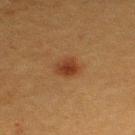Clinical impression: Recorded during total-body skin imaging; not selected for excision or biopsy. Acquisition and patient details: A close-up tile cropped from a whole-body skin photograph, about 15 mm across. Imaged with cross-polarized lighting. Located on the back. A female subject roughly 40 years of age.